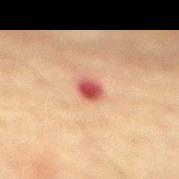Case summary:
– follow-up: catalogued during a skin exam; not biopsied